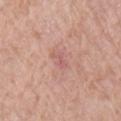The lesion was photographed on a routine skin check and not biopsied; there is no pathology result. A roughly 15 mm field-of-view crop from a total-body skin photograph. The lesion is on the arm. Imaged with white-light lighting. A male patient, approximately 80 years of age. Measured at roughly 2.5 mm in maximum diameter.